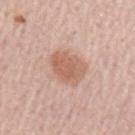Imaged during a routine full-body skin examination; the lesion was not biopsied and no histopathology is available. A roughly 15 mm field-of-view crop from a total-body skin photograph. The lesion is located on the left upper arm. A male subject, about 65 years old. Longest diameter approximately 4 mm. The tile uses white-light illumination.